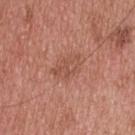Assessment: Recorded during total-body skin imaging; not selected for excision or biopsy. Context: A region of skin cropped from a whole-body photographic capture, roughly 15 mm wide. From the upper back. This is a white-light tile. The subject is a male aged 53–57. About 4 mm across.